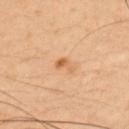Recorded during total-body skin imaging; not selected for excision or biopsy.
On the left upper arm.
The patient is a male aged 38–42.
Cropped from a whole-body photographic skin survey; the tile spans about 15 mm.